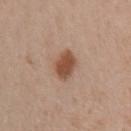Clinical impression:
Part of a total-body skin-imaging series; this lesion was reviewed on a skin check and was not flagged for biopsy.